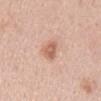| key | value |
|---|---|
| biopsy status | imaged on a skin check; not biopsied |
| diameter | ~3.5 mm (longest diameter) |
| illumination | white-light illumination |
| body site | the mid back |
| patient | male, aged 38–42 |
| image | ~15 mm tile from a whole-body skin photo |
| TBP lesion metrics | a footprint of about 5 mm² and a shape-asymmetry score of about 0.35 (0 = symmetric); a nevus-likeness score of about 90/100 and a lesion-detection confidence of about 100/100 |